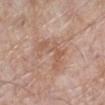Q: Is there a histopathology result?
A: catalogued during a skin exam; not biopsied
Q: How was the tile lit?
A: white-light illumination
Q: What is the anatomic site?
A: the left lower leg
Q: How large is the lesion?
A: about 5 mm
Q: What are the patient's age and sex?
A: female, aged around 70
Q: Automated lesion metrics?
A: a footprint of about 9.5 mm² and a shape eccentricity near 0.8; a border-irregularity rating of about 9/10, internal color variation of about 3.5 on a 0–10 scale, and peripheral color asymmetry of about 1
Q: What kind of image is this?
A: ~15 mm tile from a whole-body skin photo